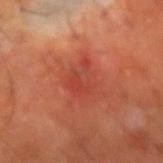Recorded during total-body skin imaging; not selected for excision or biopsy. Captured under cross-polarized illumination. On the right forearm. The recorded lesion diameter is about 3.5 mm. This image is a 15 mm lesion crop taken from a total-body photograph. The total-body-photography lesion software estimated a footprint of about 7.5 mm² and a shape-asymmetry score of about 0.4 (0 = symmetric). The software also gave a border-irregularity rating of about 5/10, a color-variation rating of about 3/10, and peripheral color asymmetry of about 1. The analysis additionally found an automated nevus-likeness rating near 5 out of 100 and a lesion-detection confidence of about 100/100. The subject is a male about 60 years old.